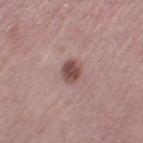notes = total-body-photography surveillance lesion; no biopsy
size = ~3 mm (longest diameter)
image source = ~15 mm crop, total-body skin-cancer survey
site = the left thigh
subject = female, aged around 55
tile lighting = white-light illumination